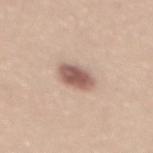Impression: No biopsy was performed on this lesion — it was imaged during a full skin examination and was not determined to be concerning. Clinical summary: The patient is a female aged 33–37. The lesion is on the mid back. A close-up tile cropped from a whole-body skin photograph, about 15 mm across. This is a white-light tile.